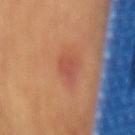patient = male, aged approximately 70 | body site = the mid back | size = about 2.5 mm | tile lighting = cross-polarized illumination | image source = 15 mm crop, total-body photography | TBP lesion metrics = a shape eccentricity near 0.75; a mean CIELAB color near L≈48 a*≈28 b*≈30 and a lesion–skin lightness drop of about 6; an automated nevus-likeness rating near 20 out of 100 and a detector confidence of about 100 out of 100 that the crop contains a lesion.